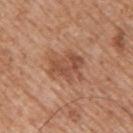The subject is a male aged around 60. A lesion tile, about 15 mm wide, cut from a 3D total-body photograph. On the back.